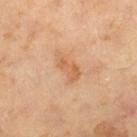Q: How was this image acquired?
A: ~15 mm crop, total-body skin-cancer survey
Q: Who is the patient?
A: male, roughly 65 years of age